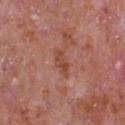Clinical impression: Imaged during a routine full-body skin examination; the lesion was not biopsied and no histopathology is available. Context: An algorithmic analysis of the crop reported a footprint of about 4.5 mm², an eccentricity of roughly 0.9, and a symmetry-axis asymmetry near 0.25. And it measured roughly 8 lightness units darker than nearby skin and a normalized lesion–skin contrast near 6.5. Located on the chest. About 3.5 mm across. The patient is a male in their mid-60s. Cropped from a whole-body photographic skin survey; the tile spans about 15 mm. Imaged with white-light lighting.